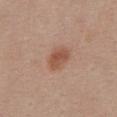Clinical impression: This lesion was catalogued during total-body skin photography and was not selected for biopsy. Acquisition and patient details: From the chest. A female subject aged 53 to 57. This image is a 15 mm lesion crop taken from a total-body photograph.